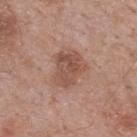automated_metrics:
  nevus_likeness_0_100: 10
  lesion_detection_confidence_0_100: 100
image:
  source: total-body photography crop
  field_of_view_mm: 15
patient:
  sex: male
  age_approx: 70
site: upper back
lighting: white-light